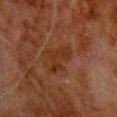Captured during whole-body skin photography for melanoma surveillance; the lesion was not biopsied.